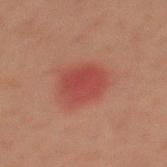A male subject about 45 years old. This is a cross-polarized tile. The lesion is located on the mid back. Measured at roughly 4 mm in maximum diameter. Cropped from a total-body skin-imaging series; the visible field is about 15 mm.